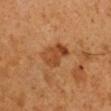The lesion was tiled from a total-body skin photograph and was not biopsied. Captured under cross-polarized illumination. An algorithmic analysis of the crop reported a footprint of about 8.5 mm² and a shape eccentricity near 0.7. It also reported a lesion-detection confidence of about 100/100. Cropped from a total-body skin-imaging series; the visible field is about 15 mm. Located on the right upper arm. The subject is a male approximately 50 years of age.